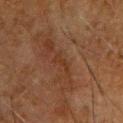biopsy_status: not biopsied; imaged during a skin examination
lighting: cross-polarized
patient:
  sex: male
  age_approx: 65
image:
  source: total-body photography crop
  field_of_view_mm: 15
site: chest
lesion_size:
  long_diameter_mm_approx: 2.5
automated_metrics:
  area_mm2_approx: 2.5
  eccentricity: 0.9
  cielab_L: 27
  cielab_a: 18
  cielab_b: 26
  vs_skin_darker_L: 5.0
  nevus_likeness_0_100: 0
  lesion_detection_confidence_0_100: 70